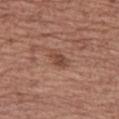follow-up: catalogued during a skin exam; not biopsied
patient: female, aged approximately 75
anatomic site: the left thigh
image: 15 mm crop, total-body photography
lesion size: about 3 mm
image-analysis metrics: an area of roughly 4 mm², a shape eccentricity near 0.8, and two-axis asymmetry of about 0.25; a mean CIELAB color near L≈46 a*≈21 b*≈27 and roughly 9 lightness units darker than nearby skin; a border-irregularity index near 2.5/10 and a color-variation rating of about 3/10; a lesion-detection confidence of about 100/100